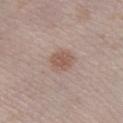Located on the right lower leg.
Captured under white-light illumination.
Measured at roughly 3.5 mm in maximum diameter.
An algorithmic analysis of the crop reported a lesion area of about 7.5 mm², a shape eccentricity near 0.5, and a symmetry-axis asymmetry near 0.2. The analysis additionally found a border-irregularity index near 2.5/10, internal color variation of about 2 on a 0–10 scale, and radial color variation of about 0.5. It also reported an automated nevus-likeness rating near 85 out of 100 and lesion-presence confidence of about 100/100.
A female subject aged 23–27.
A 15 mm close-up tile from a total-body photography series done for melanoma screening.